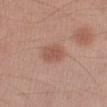{
  "biopsy_status": "not biopsied; imaged during a skin examination",
  "image": {
    "source": "total-body photography crop",
    "field_of_view_mm": 15
  },
  "patient": {
    "sex": "male",
    "age_approx": 45
  },
  "site": "left forearm"
}